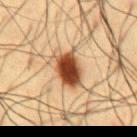Impression: Captured during whole-body skin photography for melanoma surveillance; the lesion was not biopsied. Context: On the chest. The lesion's longest dimension is about 4.5 mm. A roughly 15 mm field-of-view crop from a total-body skin photograph. The subject is a male in their 60s.